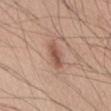Notes:
- biopsy status — catalogued during a skin exam; not biopsied
- lighting — white-light
- location — the abdomen
- subject — male, about 60 years old
- imaging modality — total-body-photography crop, ~15 mm field of view
- image-analysis metrics — an area of roughly 5 mm² and a shape-asymmetry score of about 0.2 (0 = symmetric); a border-irregularity rating of about 2.5/10, a within-lesion color-variation index near 2.5/10, and peripheral color asymmetry of about 1
- lesion diameter — ≈4 mm